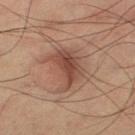This lesion was catalogued during total-body skin photography and was not selected for biopsy. This image is a 15 mm lesion crop taken from a total-body photograph. Captured under cross-polarized illumination. The patient is a male aged around 60. The total-body-photography lesion software estimated an outline eccentricity of about 0.65 (0 = round, 1 = elongated) and a symmetry-axis asymmetry near 0.4. The lesion's longest dimension is about 5 mm. Located on the left thigh.